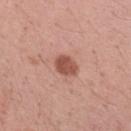Assessment:
Part of a total-body skin-imaging series; this lesion was reviewed on a skin check and was not flagged for biopsy.
Acquisition and patient details:
The tile uses white-light illumination. An algorithmic analysis of the crop reported a mean CIELAB color near L≈52 a*≈25 b*≈28, roughly 13 lightness units darker than nearby skin, and a normalized lesion–skin contrast near 9. It also reported a color-variation rating of about 2.5/10 and radial color variation of about 1. The software also gave an automated nevus-likeness rating near 85 out of 100 and a detector confidence of about 100 out of 100 that the crop contains a lesion. A region of skin cropped from a whole-body photographic capture, roughly 15 mm wide. Longest diameter approximately 3 mm. A female subject, aged 33 to 37. From the right upper arm.